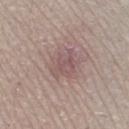{"biopsy_status": "not biopsied; imaged during a skin examination", "lighting": "white-light", "site": "left lower leg", "lesion_size": {"long_diameter_mm_approx": 3.5}, "patient": {"sex": "female", "age_approx": 60}, "image": {"source": "total-body photography crop", "field_of_view_mm": 15}, "automated_metrics": {"cielab_L": 53, "cielab_a": 18, "cielab_b": 18, "vs_skin_darker_L": 7.0, "vs_skin_contrast_norm": 5.5, "border_irregularity_0_10": 3.5, "color_variation_0_10": 2.5, "peripheral_color_asymmetry": 1.0, "nevus_likeness_0_100": 0, "lesion_detection_confidence_0_100": 95}}